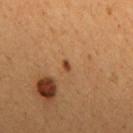Assessment:
Imaged during a routine full-body skin examination; the lesion was not biopsied and no histopathology is available.
Clinical summary:
A male subject aged around 45. Captured under cross-polarized illumination. Cropped from a whole-body photographic skin survey; the tile spans about 15 mm. Longest diameter approximately 1.5 mm. The lesion-visualizer software estimated an area of roughly 1 mm², an outline eccentricity of about 0.8 (0 = round, 1 = elongated), and a shape-asymmetry score of about 0.35 (0 = symmetric). The analysis additionally found a mean CIELAB color near L≈40 a*≈23 b*≈35, a lesion–skin lightness drop of about 12, and a lesion-to-skin contrast of about 9.5 (normalized; higher = more distinct). And it measured border irregularity of about 2.5 on a 0–10 scale and a peripheral color-asymmetry measure near 0. The lesion is located on the upper back.